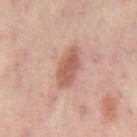Recorded during total-body skin imaging; not selected for excision or biopsy.
Imaged with cross-polarized lighting.
An algorithmic analysis of the crop reported two-axis asymmetry of about 0.15. The analysis additionally found a lesion color around L≈56 a*≈24 b*≈28 in CIELAB, a lesion–skin lightness drop of about 11, and a normalized lesion–skin contrast near 8.
The lesion's longest dimension is about 4.5 mm.
A 15 mm close-up extracted from a 3D total-body photography capture.
A patient in their mid- to late 50s.
On the mid back.